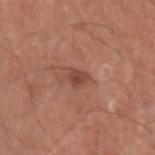<case>
<biopsy_status>not biopsied; imaged during a skin examination</biopsy_status>
<image>
  <source>total-body photography crop</source>
  <field_of_view_mm>15</field_of_view_mm>
</image>
<automated_metrics>
  <area_mm2_approx>3.5</area_mm2_approx>
  <eccentricity>0.7</eccentricity>
  <vs_skin_darker_L>9.0</vs_skin_darker_L>
  <vs_skin_contrast_norm>7.0</vs_skin_contrast_norm>
  <nevus_likeness_0_100>40</nevus_likeness_0_100>
  <lesion_detection_confidence_0_100>100</lesion_detection_confidence_0_100>
</automated_metrics>
<lesion_size>
  <long_diameter_mm_approx>2.5</long_diameter_mm_approx>
</lesion_size>
<lighting>white-light</lighting>
<site>left upper arm</site>
<patient>
  <sex>male</sex>
  <age_approx>65</age_approx>
</patient>
</case>